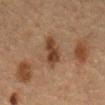biopsy status: no biopsy performed (imaged during a skin exam) | image source: total-body-photography crop, ~15 mm field of view | subject: male, roughly 65 years of age | automated lesion analysis: a lesion color around L≈34 a*≈17 b*≈27 in CIELAB and a normalized border contrast of about 9; a classifier nevus-likeness of about 5/100 and a detector confidence of about 100 out of 100 that the crop contains a lesion | anatomic site: the abdomen.